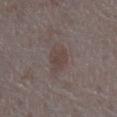<tbp_lesion>
  <biopsy_status>not biopsied; imaged during a skin examination</biopsy_status>
  <lesion_size>
    <long_diameter_mm_approx>3.0</long_diameter_mm_approx>
  </lesion_size>
  <patient>
    <sex>male</sex>
    <age_approx>65</age_approx>
  </patient>
  <lighting>white-light</lighting>
  <site>abdomen</site>
  <image>
    <source>total-body photography crop</source>
    <field_of_view_mm>15</field_of_view_mm>
  </image>
</tbp_lesion>